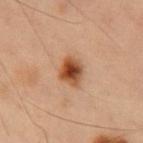biopsy status=total-body-photography surveillance lesion; no biopsy
lesion diameter=≈3 mm
site=the front of the torso
image source=total-body-photography crop, ~15 mm field of view
patient=male, aged 53–57
illumination=cross-polarized illumination
TBP lesion metrics=a border-irregularity rating of about 1.5/10 and a within-lesion color-variation index near 7.5/10; a nevus-likeness score of about 100/100 and a detector confidence of about 100 out of 100 that the crop contains a lesion